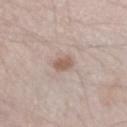biopsy status: catalogued during a skin exam; not biopsied
site: the arm
diameter: about 3 mm
lighting: white-light
image: 15 mm crop, total-body photography
subject: male, aged 68 to 72